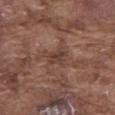Clinical impression: Imaged during a routine full-body skin examination; the lesion was not biopsied and no histopathology is available.